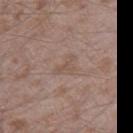{"biopsy_status": "not biopsied; imaged during a skin examination", "lighting": "white-light", "site": "right thigh", "image": {"source": "total-body photography crop", "field_of_view_mm": 15}, "patient": {"sex": "male", "age_approx": 45}}